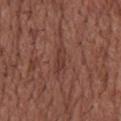| key | value |
|---|---|
| patient | male, aged 63–67 |
| size | ≈3.5 mm |
| illumination | white-light illumination |
| site | the head or neck |
| automated metrics | a lesion color around L≈37 a*≈22 b*≈24 in CIELAB and a normalized lesion–skin contrast near 5.5; a border-irregularity rating of about 2.5/10 and peripheral color asymmetry of about 0.5; a classifier nevus-likeness of about 0/100 and a lesion-detection confidence of about 90/100 |
| image source | 15 mm crop, total-body photography |